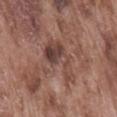The lesion was photographed on a routine skin check and not biopsied; there is no pathology result. The total-body-photography lesion software estimated an area of roughly 12 mm². The analysis additionally found a border-irregularity index near 6.5/10, internal color variation of about 7.5 on a 0–10 scale, and peripheral color asymmetry of about 3. The software also gave a nevus-likeness score of about 5/100. Longest diameter approximately 5.5 mm. A male patient aged 73 to 77. A roughly 15 mm field-of-view crop from a total-body skin photograph. Captured under white-light illumination. Located on the lower back.